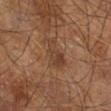site — the leg | acquisition — total-body-photography crop, ~15 mm field of view | illumination — cross-polarized | patient — male, approximately 60 years of age | TBP lesion metrics — a border-irregularity rating of about 4/10, internal color variation of about 3 on a 0–10 scale, and peripheral color asymmetry of about 1; lesion-presence confidence of about 100/100.